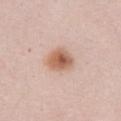workup — no biopsy performed (imaged during a skin exam); subject — female, about 40 years old; size — ~3.5 mm (longest diameter); tile lighting — white-light; anatomic site — the abdomen; image source — 15 mm crop, total-body photography.